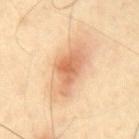Part of a total-body skin-imaging series; this lesion was reviewed on a skin check and was not flagged for biopsy. A close-up tile cropped from a whole-body skin photograph, about 15 mm across. About 7.5 mm across. Imaged with cross-polarized lighting. From the mid back. The patient is a male aged 63 to 67. Automated image analysis of the tile measured a shape eccentricity near 0.9 and a shape-asymmetry score of about 0.2 (0 = symmetric).